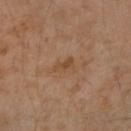{
  "biopsy_status": "not biopsied; imaged during a skin examination",
  "lesion_size": {
    "long_diameter_mm_approx": 2.5
  },
  "site": "right upper arm",
  "image": {
    "source": "total-body photography crop",
    "field_of_view_mm": 15
  },
  "lighting": "cross-polarized",
  "patient": {
    "sex": "female",
    "age_approx": 45
  }
}